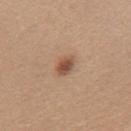follow-up — total-body-photography surveillance lesion; no biopsy | body site — the mid back | image — ~15 mm tile from a whole-body skin photo | automated lesion analysis — a lesion area of about 4 mm², an outline eccentricity of about 0.7 (0 = round, 1 = elongated), and two-axis asymmetry of about 0.25; border irregularity of about 2.5 on a 0–10 scale, a color-variation rating of about 3.5/10, and a peripheral color-asymmetry measure near 1.5 | patient — female, in their mid-50s | lighting — white-light illumination.